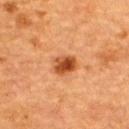Assessment: The lesion was photographed on a routine skin check and not biopsied; there is no pathology result. Clinical summary: A close-up tile cropped from a whole-body skin photograph, about 15 mm across. The lesion is located on the upper back. About 3 mm across. A female patient about 55 years old. The tile uses cross-polarized illumination.